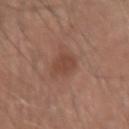Q: Was this lesion biopsied?
A: catalogued during a skin exam; not biopsied
Q: What lighting was used for the tile?
A: white-light
Q: Patient demographics?
A: male, approximately 40 years of age
Q: What did automated image analysis measure?
A: a mean CIELAB color near L≈44 a*≈22 b*≈27; radial color variation of about 0.5
Q: Where on the body is the lesion?
A: the right forearm
Q: How large is the lesion?
A: ≈3 mm
Q: How was this image acquired?
A: total-body-photography crop, ~15 mm field of view